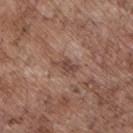Q: Was a biopsy performed?
A: no biopsy performed (imaged during a skin exam)
Q: What are the patient's age and sex?
A: male, roughly 70 years of age
Q: How large is the lesion?
A: ~3 mm (longest diameter)
Q: What kind of image is this?
A: ~15 mm tile from a whole-body skin photo
Q: Automated lesion metrics?
A: a border-irregularity rating of about 4/10, a color-variation rating of about 1/10, and a peripheral color-asymmetry measure near 0.5; a classifier nevus-likeness of about 0/100
Q: Lesion location?
A: the chest
Q: Illumination type?
A: white-light